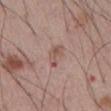Clinical impression:
Recorded during total-body skin imaging; not selected for excision or biopsy.
Acquisition and patient details:
A roughly 15 mm field-of-view crop from a total-body skin photograph. The lesion-visualizer software estimated a lesion color around L≈53 a*≈20 b*≈24 in CIELAB. Captured under white-light illumination. The recorded lesion diameter is about 3.5 mm. The lesion is on the abdomen. A male patient aged 53 to 57.